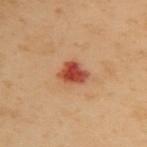notes: total-body-photography surveillance lesion; no biopsy
subject: female, aged approximately 60
acquisition: ~15 mm tile from a whole-body skin photo
anatomic site: the back